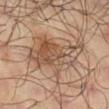follow-up: total-body-photography surveillance lesion; no biopsy
subject: male, aged around 65
acquisition: ~15 mm crop, total-body skin-cancer survey
location: the leg